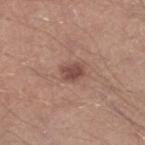This lesion was catalogued during total-body skin photography and was not selected for biopsy.